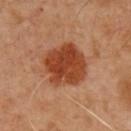<tbp_lesion>
<biopsy_status>not biopsied; imaged during a skin examination</biopsy_status>
<lesion_size>
  <long_diameter_mm_approx>5.0</long_diameter_mm_approx>
</lesion_size>
<patient>
  <sex>male</sex>
  <age_approx>50</age_approx>
</patient>
<image>
  <source>total-body photography crop</source>
  <field_of_view_mm>15</field_of_view_mm>
</image>
<site>chest</site>
</tbp_lesion>